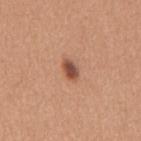| feature | finding |
|---|---|
| biopsy status | imaged on a skin check; not biopsied |
| site | the mid back |
| image | ~15 mm tile from a whole-body skin photo |
| subject | female, about 45 years old |